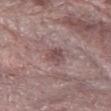Q: Was this lesion biopsied?
A: imaged on a skin check; not biopsied
Q: What is the imaging modality?
A: ~15 mm tile from a whole-body skin photo
Q: Who is the patient?
A: female, aged around 65
Q: What is the lesion's diameter?
A: about 3 mm
Q: What lighting was used for the tile?
A: white-light
Q: Automated lesion metrics?
A: an eccentricity of roughly 0.7 and two-axis asymmetry of about 0.2; an average lesion color of about L≈48 a*≈18 b*≈18 (CIELAB), roughly 9 lightness units darker than nearby skin, and a normalized border contrast of about 7
Q: Lesion location?
A: the right forearm